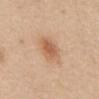<tbp_lesion>
<biopsy_status>not biopsied; imaged during a skin examination</biopsy_status>
<site>abdomen</site>
<image>
  <source>total-body photography crop</source>
  <field_of_view_mm>15</field_of_view_mm>
</image>
<patient>
  <sex>female</sex>
  <age_approx>70</age_approx>
</patient>
</tbp_lesion>